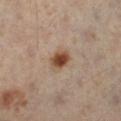Assessment: The lesion was tiled from a total-body skin photograph and was not biopsied. Background: From the leg. The tile uses cross-polarized illumination. Cropped from a whole-body photographic skin survey; the tile spans about 15 mm. Approximately 3 mm at its widest. A male patient, aged 48 to 52.